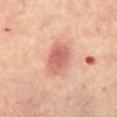<record>
<biopsy_status>not biopsied; imaged during a skin examination</biopsy_status>
<site>right leg</site>
<image>
  <source>total-body photography crop</source>
  <field_of_view_mm>15</field_of_view_mm>
</image>
<lesion_size>
  <long_diameter_mm_approx>4.0</long_diameter_mm_approx>
</lesion_size>
<automated_metrics>
  <border_irregularity_0_10>1.5</border_irregularity_0_10>
  <color_variation_0_10>4.0</color_variation_0_10>
  <nevus_likeness_0_100>80</nevus_likeness_0_100>
  <lesion_detection_confidence_0_100>100</lesion_detection_confidence_0_100>
</automated_metrics>
<patient>
  <sex>female</sex>
  <age_approx>65</age_approx>
</patient>
</record>